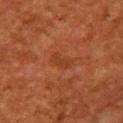imaging modality — 15 mm crop, total-body photography; subject — female, in their 50s; site — the chest.